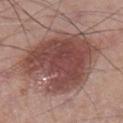Part of a total-body skin-imaging series; this lesion was reviewed on a skin check and was not flagged for biopsy. The subject is a male in their mid- to late 50s. From the left lower leg. A close-up tile cropped from a whole-body skin photograph, about 15 mm across. Approximately 9.5 mm at its widest. Automated tile analysis of the lesion measured a within-lesion color-variation index near 4.5/10. This is a white-light tile.